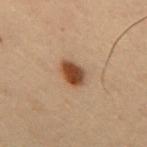biopsy_status: not biopsied; imaged during a skin examination
patient:
  sex: male
  age_approx: 55
image:
  source: total-body photography crop
  field_of_view_mm: 15
lighting: cross-polarized
site: mid back